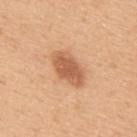biopsy status = total-body-photography surveillance lesion; no biopsy
body site = the back
patient = male, approximately 50 years of age
acquisition = ~15 mm tile from a whole-body skin photo
lighting = white-light illumination